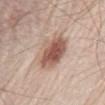This lesion was catalogued during total-body skin photography and was not selected for biopsy. An algorithmic analysis of the crop reported a footprint of about 14 mm², an outline eccentricity of about 0.7 (0 = round, 1 = elongated), and two-axis asymmetry of about 0.15. The analysis additionally found a border-irregularity index near 2/10, internal color variation of about 6.5 on a 0–10 scale, and a peripheral color-asymmetry measure near 2. The analysis additionally found a classifier nevus-likeness of about 95/100 and a detector confidence of about 100 out of 100 that the crop contains a lesion. The patient is a male aged approximately 80. This is a white-light tile. Approximately 5 mm at its widest. Cropped from a total-body skin-imaging series; the visible field is about 15 mm. On the abdomen.